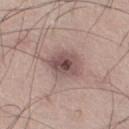| field | value |
|---|---|
| follow-up | catalogued during a skin exam; not biopsied |
| TBP lesion metrics | a shape eccentricity near 0.6 and a symmetry-axis asymmetry near 0.15; a classifier nevus-likeness of about 30/100 and lesion-presence confidence of about 100/100 |
| subject | male, aged 28 to 32 |
| size | ≈4 mm |
| anatomic site | the leg |
| lighting | white-light illumination |
| image | total-body-photography crop, ~15 mm field of view |